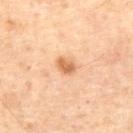follow-up=no biopsy performed (imaged during a skin exam) | automated lesion analysis=an area of roughly 4 mm², a shape eccentricity near 0.7, and two-axis asymmetry of about 0.2; a border-irregularity index near 1.5/10 and a peripheral color-asymmetry measure near 0.5 | image source=15 mm crop, total-body photography | lighting=cross-polarized illumination | subject=male, aged 68–72 | size=≈2.5 mm | site=the abdomen.